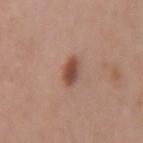Impression:
Captured during whole-body skin photography for melanoma surveillance; the lesion was not biopsied.
Clinical summary:
A 15 mm close-up extracted from a 3D total-body photography capture. From the right forearm. Captured under white-light illumination. About 3 mm across. The patient is a female about 35 years old.